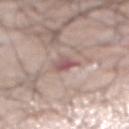The lesion was tiled from a total-body skin photograph and was not biopsied. Automated image analysis of the tile measured an automated nevus-likeness rating near 0 out of 100 and a detector confidence of about 95 out of 100 that the crop contains a lesion. A region of skin cropped from a whole-body photographic capture, roughly 15 mm wide. The lesion is on the abdomen. The tile uses white-light illumination. A male patient aged 58–62. Longest diameter approximately 3 mm.